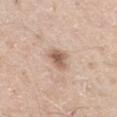Impression: The lesion was tiled from a total-body skin photograph and was not biopsied. Acquisition and patient details: The lesion is on the right thigh. Cropped from a whole-body photographic skin survey; the tile spans about 15 mm. The lesion-visualizer software estimated a lesion area of about 5 mm², a shape eccentricity near 0.45, and two-axis asymmetry of about 0.25. And it measured a border-irregularity index near 2.5/10, internal color variation of about 3.5 on a 0–10 scale, and radial color variation of about 1. It also reported a classifier nevus-likeness of about 70/100 and a detector confidence of about 100 out of 100 that the crop contains a lesion. This is a white-light tile. The patient is a male aged around 60. Longest diameter approximately 2.5 mm.